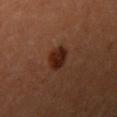The lesion was photographed on a routine skin check and not biopsied; there is no pathology result. The lesion-visualizer software estimated an outline eccentricity of about 0.55 (0 = round, 1 = elongated) and two-axis asymmetry of about 0.2. It also reported a mean CIELAB color near L≈23 a*≈22 b*≈26 and roughly 11 lightness units darker than nearby skin. It also reported a border-irregularity rating of about 2/10, a within-lesion color-variation index near 3.5/10, and peripheral color asymmetry of about 1. The lesion is on the right upper arm. A 15 mm close-up tile from a total-body photography series done for melanoma screening. The lesion's longest dimension is about 3 mm. A female patient aged around 35. Imaged with cross-polarized lighting.